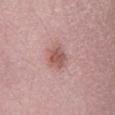Background:
Captured under white-light illumination. The lesion is on the left lower leg. A roughly 15 mm field-of-view crop from a total-body skin photograph. The subject is a female aged 38 to 42. The recorded lesion diameter is about 3 mm.